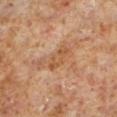Q: Was a biopsy performed?
A: total-body-photography surveillance lesion; no biopsy
Q: What is the imaging modality?
A: ~15 mm crop, total-body skin-cancer survey
Q: Patient demographics?
A: male, about 60 years old
Q: Where on the body is the lesion?
A: the left lower leg
Q: What did automated image analysis measure?
A: border irregularity of about 5.5 on a 0–10 scale, a color-variation rating of about 1/10, and radial color variation of about 0
Q: Illumination type?
A: cross-polarized illumination
Q: Lesion size?
A: about 3.5 mm